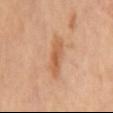workup=imaged on a skin check; not biopsied
image source=15 mm crop, total-body photography
lighting=cross-polarized illumination
site=the mid back
lesion size=~4.5 mm (longest diameter)